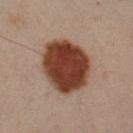Impression:
Recorded during total-body skin imaging; not selected for excision or biopsy.
Clinical summary:
A lesion tile, about 15 mm wide, cut from a 3D total-body photograph. The patient is a male aged around 50. The lesion is located on the left forearm.